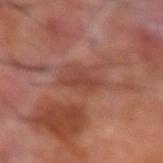The subject is a male aged 68 to 72. A roughly 15 mm field-of-view crop from a total-body skin photograph. The lesion is on the right forearm.